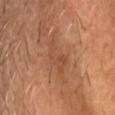Findings:
– body site · the head or neck
– image · ~15 mm tile from a whole-body skin photo
– tile lighting · cross-polarized
– subject · male, aged 68–72
– automated lesion analysis · a footprint of about 12 mm², a shape eccentricity near 0.95, and a symmetry-axis asymmetry near 0.35; an average lesion color of about L≈48 a*≈22 b*≈31 (CIELAB)